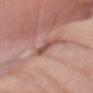– biopsy status — total-body-photography surveillance lesion; no biopsy
– body site — the left forearm
– subject — female, roughly 75 years of age
– image-analysis metrics — an eccentricity of roughly 0.4 and two-axis asymmetry of about 0.3; a mean CIELAB color near L≈53 a*≈23 b*≈25, roughly 10 lightness units darker than nearby skin, and a normalized border contrast of about 7; internal color variation of about 4 on a 0–10 scale and a peripheral color-asymmetry measure near 1; a lesion-detection confidence of about 95/100
– image source — total-body-photography crop, ~15 mm field of view